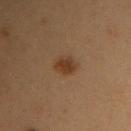Impression: The lesion was photographed on a routine skin check and not biopsied; there is no pathology result. Background: The subject is a female in their 40s. The tile uses cross-polarized illumination. The recorded lesion diameter is about 3 mm. A lesion tile, about 15 mm wide, cut from a 3D total-body photograph. The lesion is located on the arm.